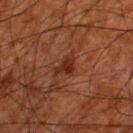Part of a total-body skin-imaging series; this lesion was reviewed on a skin check and was not flagged for biopsy.